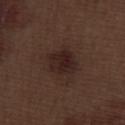biopsy_status: not biopsied; imaged during a skin examination
site: leg
patient:
  sex: male
  age_approx: 70
image:
  source: total-body photography crop
  field_of_view_mm: 15
automated_metrics:
  cielab_L: 22
  cielab_a: 16
  cielab_b: 18
  vs_skin_darker_L: 7.0
  vs_skin_contrast_norm: 9.0
  color_variation_0_10: 4.0
  peripheral_color_asymmetry: 1.5